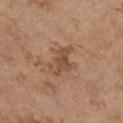Captured during whole-body skin photography for melanoma surveillance; the lesion was not biopsied.
A female subject, aged approximately 65.
From the chest.
A 15 mm close-up tile from a total-body photography series done for melanoma screening.